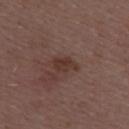{"patient": {"sex": "female", "age_approx": 45}, "automated_metrics": {"cielab_L": 34, "cielab_a": 18, "cielab_b": 22, "vs_skin_darker_L": 8.0, "vs_skin_contrast_norm": 7.5, "lesion_detection_confidence_0_100": 100}, "image": {"source": "total-body photography crop", "field_of_view_mm": 15}, "lighting": "white-light", "site": "upper back"}